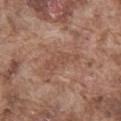The lesion was tiled from a total-body skin photograph and was not biopsied.
This is a white-light tile.
The lesion is on the front of the torso.
About 4.5 mm across.
The lesion-visualizer software estimated a footprint of about 6 mm², a shape eccentricity near 0.95, and two-axis asymmetry of about 0.35.
Cropped from a total-body skin-imaging series; the visible field is about 15 mm.
A male patient, aged approximately 75.